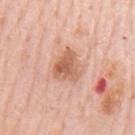{"biopsy_status": "not biopsied; imaged during a skin examination", "site": "mid back", "patient": {"sex": "male", "age_approx": 80}, "image": {"source": "total-body photography crop", "field_of_view_mm": 15}}